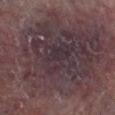{"lesion_size": {"long_diameter_mm_approx": 19.0}, "patient": {"sex": "male", "age_approx": 60}, "automated_metrics": {"eccentricity": 0.8, "shape_asymmetry": 0.3, "border_irregularity_0_10": 7.5, "color_variation_0_10": 5.0, "nevus_likeness_0_100": 0, "lesion_detection_confidence_0_100": 80}, "lighting": "cross-polarized", "image": {"source": "total-body photography crop", "field_of_view_mm": 15}, "site": "right lower leg"}